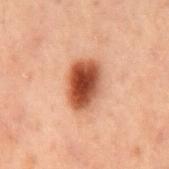Recorded during total-body skin imaging; not selected for excision or biopsy. Cropped from a total-body skin-imaging series; the visible field is about 15 mm. Approximately 5 mm at its widest. The subject is a male roughly 60 years of age. This is a cross-polarized tile. From the mid back.